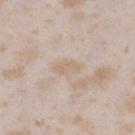Q: Was a biopsy performed?
A: catalogued during a skin exam; not biopsied
Q: Illumination type?
A: white-light
Q: What is the imaging modality?
A: 15 mm crop, total-body photography
Q: What is the anatomic site?
A: the left lower leg
Q: What is the lesion's diameter?
A: ~3.5 mm (longest diameter)
Q: What are the patient's age and sex?
A: female, in their mid- to late 20s